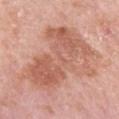<lesion>
<biopsy_status>not biopsied; imaged during a skin examination</biopsy_status>
<automated_metrics>
  <nevus_likeness_0_100>0</nevus_likeness_0_100>
  <lesion_detection_confidence_0_100>100</lesion_detection_confidence_0_100>
</automated_metrics>
<patient>
  <sex>female</sex>
  <age_approx>65</age_approx>
</patient>
<site>right upper arm</site>
<lesion_size>
  <long_diameter_mm_approx>10.5</long_diameter_mm_approx>
</lesion_size>
<image>
  <source>total-body photography crop</source>
  <field_of_view_mm>15</field_of_view_mm>
</image>
</lesion>